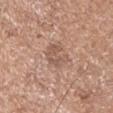{
  "biopsy_status": "not biopsied; imaged during a skin examination",
  "patient": {
    "sex": "male",
    "age_approx": 70
  },
  "site": "left upper arm",
  "lesion_size": {
    "long_diameter_mm_approx": 3.5
  },
  "image": {
    "source": "total-body photography crop",
    "field_of_view_mm": 15
  },
  "automated_metrics": {
    "area_mm2_approx": 6.0,
    "eccentricity": 0.8,
    "shape_asymmetry": 0.4,
    "cielab_L": 55,
    "cielab_a": 19,
    "cielab_b": 27,
    "vs_skin_darker_L": 8.0,
    "vs_skin_contrast_norm": 5.5
  }
}